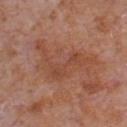Case summary:
– biopsy status · no biopsy performed (imaged during a skin exam)
– subject · male, approximately 65 years of age
– body site · the chest
– size · about 8 mm
– imaging modality · 15 mm crop, total-body photography
– lighting · white-light